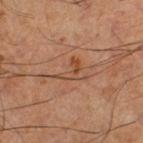Q: Was this lesion biopsied?
A: no biopsy performed (imaged during a skin exam)